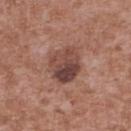Imaged during a routine full-body skin examination; the lesion was not biopsied and no histopathology is available.
A male subject, aged 43–47.
Cropped from a total-body skin-imaging series; the visible field is about 15 mm.
Located on the upper back.